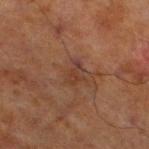Part of a total-body skin-imaging series; this lesion was reviewed on a skin check and was not flagged for biopsy.
Located on the left lower leg.
The lesion's longest dimension is about 3 mm.
A 15 mm close-up tile from a total-body photography series done for melanoma screening.
A male patient approximately 70 years of age.
Imaged with cross-polarized lighting.
The lesion-visualizer software estimated an eccentricity of roughly 0.85 and a symmetry-axis asymmetry near 0.45. The analysis additionally found a mean CIELAB color near L≈30 a*≈18 b*≈24, about 6 CIELAB-L* units darker than the surrounding skin, and a lesion-to-skin contrast of about 6 (normalized; higher = more distinct).